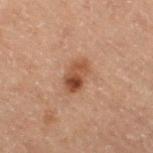Assessment:
The lesion was tiled from a total-body skin photograph and was not biopsied.
Acquisition and patient details:
The lesion's longest dimension is about 3.5 mm. A male patient aged around 70. A 15 mm crop from a total-body photograph taken for skin-cancer surveillance. From the right thigh. Automated image analysis of the tile measured a lesion area of about 6.5 mm² and a symmetry-axis asymmetry near 0.2. And it measured an average lesion color of about L≈36 a*≈18 b*≈26 (CIELAB) and roughly 9 lightness units darker than nearby skin. And it measured a border-irregularity index near 2/10, a within-lesion color-variation index near 8/10, and peripheral color asymmetry of about 3.5. This is a cross-polarized tile.